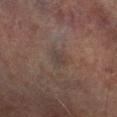Imaged during a routine full-body skin examination; the lesion was not biopsied and no histopathology is available.
A male subject aged around 75.
A roughly 15 mm field-of-view crop from a total-body skin photograph.
From the leg.
Captured under cross-polarized illumination.